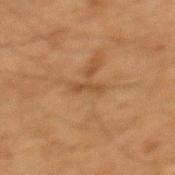Captured during whole-body skin photography for melanoma surveillance; the lesion was not biopsied. A lesion tile, about 15 mm wide, cut from a 3D total-body photograph. On the mid back. A male subject about 65 years old.